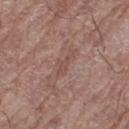<case>
  <biopsy_status>not biopsied; imaged during a skin examination</biopsy_status>
  <site>left thigh</site>
  <patient>
    <sex>female</sex>
    <age_approx>80</age_approx>
  </patient>
  <image>
    <source>total-body photography crop</source>
    <field_of_view_mm>15</field_of_view_mm>
  </image>
  <automated_metrics>
    <area_mm2_approx>3.5</area_mm2_approx>
    <eccentricity>0.9</eccentricity>
    <shape_asymmetry>0.4</shape_asymmetry>
    <nevus_likeness_0_100>0</nevus_likeness_0_100>
    <lesion_detection_confidence_0_100>85</lesion_detection_confidence_0_100>
  </automated_metrics>
</case>